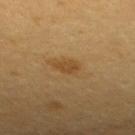Case summary:
- workup — catalogued during a skin exam; not biopsied
- anatomic site — the upper back
- acquisition — ~15 mm crop, total-body skin-cancer survey
- patient — female, approximately 50 years of age